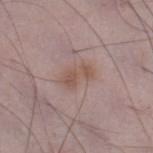notes=total-body-photography surveillance lesion; no biopsy
tile lighting=white-light illumination
location=the leg
image source=~15 mm tile from a whole-body skin photo
lesion size=~3.5 mm (longest diameter)
patient=male, aged approximately 50